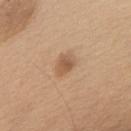Findings:
* biopsy status — total-body-photography surveillance lesion; no biopsy
* body site — the upper back
* diameter — ≈2.5 mm
* imaging modality — ~15 mm tile from a whole-body skin photo
* tile lighting — white-light
* subject — male, about 60 years old